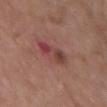Case summary:
• biopsy status · no biopsy performed (imaged during a skin exam)
• patient · female, aged around 65
• size · ≈4.5 mm
• anatomic site · the chest
• illumination · white-light
• imaging modality · 15 mm crop, total-body photography